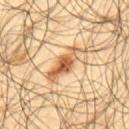Impression:
Imaged during a routine full-body skin examination; the lesion was not biopsied and no histopathology is available.
Image and clinical context:
A male patient, approximately 65 years of age. The lesion is located on the upper back. A 15 mm close-up extracted from a 3D total-body photography capture.